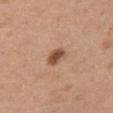Q: Is there a histopathology result?
A: catalogued during a skin exam; not biopsied
Q: What kind of image is this?
A: 15 mm crop, total-body photography
Q: Automated lesion metrics?
A: a lesion area of about 4 mm², an eccentricity of roughly 0.75, and a symmetry-axis asymmetry near 0.15; a lesion color around L≈50 a*≈22 b*≈31 in CIELAB, roughly 14 lightness units darker than nearby skin, and a normalized lesion–skin contrast near 10; a lesion-detection confidence of about 100/100
Q: Who is the patient?
A: female, aged 38 to 42
Q: How large is the lesion?
A: ~2.5 mm (longest diameter)
Q: How was the tile lit?
A: white-light
Q: What is the anatomic site?
A: the left upper arm